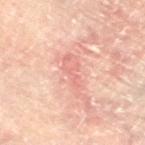{
  "biopsy_status": "not biopsied; imaged during a skin examination",
  "lighting": "cross-polarized",
  "site": "left lower leg",
  "patient": {
    "sex": "male",
    "age_approx": 60
  },
  "image": {
    "source": "total-body photography crop",
    "field_of_view_mm": 15
  }
}